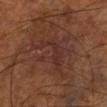biopsy status: catalogued during a skin exam; not biopsied | lesion diameter: about 6 mm | subject: male, in their 70s | image: 15 mm crop, total-body photography | site: the left lower leg | image-analysis metrics: a lesion area of about 13 mm²; a border-irregularity rating of about 9/10, a color-variation rating of about 2.5/10, and radial color variation of about 0.5 | tile lighting: cross-polarized.